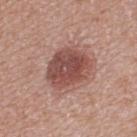Notes:
* biopsy status · imaged on a skin check; not biopsied
* imaging modality · ~15 mm crop, total-body skin-cancer survey
* subject · female, aged 38 to 42
* site · the upper back